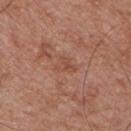lesion diameter — about 3 mm
illumination — white-light illumination
imaging modality — ~15 mm crop, total-body skin-cancer survey
patient — male, in their mid-50s
anatomic site — the chest